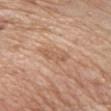Case summary:
- biopsy status · total-body-photography surveillance lesion; no biopsy
- acquisition · ~15 mm tile from a whole-body skin photo
- site · the chest
- illumination · white-light illumination
- patient · female, aged 68 to 72